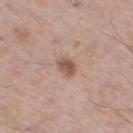<lesion>
  <biopsy_status>not biopsied; imaged during a skin examination</biopsy_status>
  <patient>
    <sex>male</sex>
    <age_approx>55</age_approx>
  </patient>
  <site>right thigh</site>
  <image>
    <source>total-body photography crop</source>
    <field_of_view_mm>15</field_of_view_mm>
  </image>
  <lesion_size>
    <long_diameter_mm_approx>3.0</long_diameter_mm_approx>
  </lesion_size>
  <lighting>white-light</lighting>
</lesion>